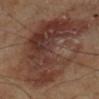Q: Was this lesion biopsied?
A: catalogued during a skin exam; not biopsied
Q: Who is the patient?
A: male, about 70 years old
Q: What is the lesion's diameter?
A: ~11 mm (longest diameter)
Q: What kind of image is this?
A: ~15 mm tile from a whole-body skin photo
Q: What did automated image analysis measure?
A: a border-irregularity rating of about 6.5/10, a within-lesion color-variation index near 6.5/10, and a peripheral color-asymmetry measure near 2; an automated nevus-likeness rating near 0 out of 100 and a lesion-detection confidence of about 100/100
Q: Lesion location?
A: the right lower leg